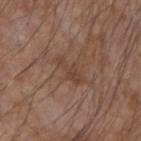Assessment:
No biopsy was performed on this lesion — it was imaged during a full skin examination and was not determined to be concerning.
Background:
A male patient aged 73–77. Located on the left forearm. Measured at roughly 4 mm in maximum diameter. This image is a 15 mm lesion crop taken from a total-body photograph. This is a white-light tile.